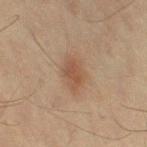biopsy status: catalogued during a skin exam; not biopsied
subject: female, in their mid-50s
illumination: cross-polarized illumination
anatomic site: the left thigh
image source: ~15 mm crop, total-body skin-cancer survey
lesion diameter: ≈4 mm